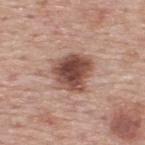Recorded during total-body skin imaging; not selected for excision or biopsy.